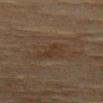{"biopsy_status": "not biopsied; imaged during a skin examination", "patient": {"sex": "female", "age_approx": 80}, "image": {"source": "total-body photography crop", "field_of_view_mm": 15}, "automated_metrics": {"area_mm2_approx": 5.5, "eccentricity": 0.75, "shape_asymmetry": 0.4, "border_irregularity_0_10": 3.5, "color_variation_0_10": 2.0}, "lighting": "cross-polarized", "site": "back", "lesion_size": {"long_diameter_mm_approx": 3.5}}